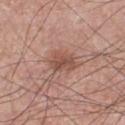subject: male, in their mid-50s; body site: the chest; acquisition: ~15 mm crop, total-body skin-cancer survey.